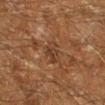The lesion was photographed on a routine skin check and not biopsied; there is no pathology result.